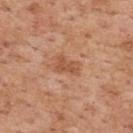Recorded during total-body skin imaging; not selected for excision or biopsy. A male subject aged around 60. An algorithmic analysis of the crop reported a lesion area of about 4.5 mm², an outline eccentricity of about 0.8 (0 = round, 1 = elongated), and a symmetry-axis asymmetry near 0.35. And it measured an automated nevus-likeness rating near 0 out of 100 and lesion-presence confidence of about 100/100. The tile uses white-light illumination. The lesion's longest dimension is about 3 mm. A 15 mm close-up extracted from a 3D total-body photography capture. On the back.